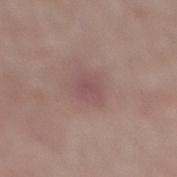Assessment:
This lesion was catalogued during total-body skin photography and was not selected for biopsy.
Context:
The patient is a male approximately 70 years of age. A roughly 15 mm field-of-view crop from a total-body skin photograph. The lesion is located on the mid back. The tile uses white-light illumination. An algorithmic analysis of the crop reported a within-lesion color-variation index near 2/10. And it measured an automated nevus-likeness rating near 0 out of 100 and a lesion-detection confidence of about 100/100.